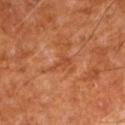body site — the left upper arm
image source — total-body-photography crop, ~15 mm field of view
lighting — cross-polarized illumination
subject — male, in their mid- to late 60s
automated metrics — a footprint of about 3 mm², a shape eccentricity near 0.85, and a shape-asymmetry score of about 0.5 (0 = symmetric); a border-irregularity index near 5.5/10 and a within-lesion color-variation index near 1/10; a lesion-detection confidence of about 90/100